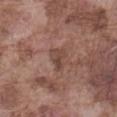Findings:
– follow-up · catalogued during a skin exam; not biopsied
– automated lesion analysis · a mean CIELAB color near L≈44 a*≈19 b*≈23, a lesion–skin lightness drop of about 8, and a lesion-to-skin contrast of about 6.5 (normalized; higher = more distinct); a border-irregularity index near 4.5/10 and a peripheral color-asymmetry measure near 0.5; an automated nevus-likeness rating near 0 out of 100 and a lesion-detection confidence of about 95/100
– acquisition · 15 mm crop, total-body photography
– patient · male, in their mid-70s
– lesion diameter · ~3 mm (longest diameter)
– lighting · white-light
– anatomic site · the abdomen